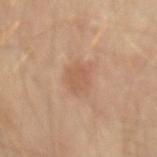The lesion was tiled from a total-body skin photograph and was not biopsied. Imaged with cross-polarized lighting. A patient in their mid-50s. The recorded lesion diameter is about 3 mm. A region of skin cropped from a whole-body photographic capture, roughly 15 mm wide. The lesion is on the left forearm.